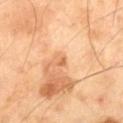Q: Was this lesion biopsied?
A: imaged on a skin check; not biopsied
Q: Where on the body is the lesion?
A: the leg
Q: How was this image acquired?
A: ~15 mm tile from a whole-body skin photo
Q: How was the tile lit?
A: cross-polarized illumination
Q: Automated lesion metrics?
A: an area of roughly 1.5 mm² and a shape-asymmetry score of about 0.3 (0 = symmetric); an average lesion color of about L≈64 a*≈25 b*≈41 (CIELAB), roughly 10 lightness units darker than nearby skin, and a lesion-to-skin contrast of about 6.5 (normalized; higher = more distinct)
Q: What are the patient's age and sex?
A: male, in their 70s
Q: How large is the lesion?
A: about 1.5 mm